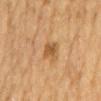follow-up: catalogued during a skin exam; not biopsied
body site: the mid back
automated metrics: a footprint of about 4 mm², an eccentricity of roughly 0.75, and a shape-asymmetry score of about 0.25 (0 = symmetric); a border-irregularity index near 2.5/10, internal color variation of about 2 on a 0–10 scale, and a peripheral color-asymmetry measure near 0.5
lesion size: ≈2.5 mm
imaging modality: 15 mm crop, total-body photography
subject: male, aged 83 or over